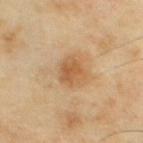Q: Was this lesion biopsied?
A: imaged on a skin check; not biopsied
Q: What lighting was used for the tile?
A: cross-polarized
Q: How was this image acquired?
A: ~15 mm crop, total-body skin-cancer survey
Q: What are the patient's age and sex?
A: male, roughly 55 years of age
Q: What is the anatomic site?
A: the chest